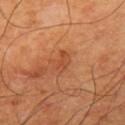| key | value |
|---|---|
| follow-up | catalogued during a skin exam; not biopsied |
| subject | male, aged around 65 |
| image source | ~15 mm crop, total-body skin-cancer survey |
| body site | the left upper arm |